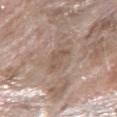notes — catalogued during a skin exam; not biopsied